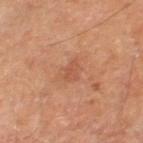follow-up: no biopsy performed (imaged during a skin exam) | TBP lesion metrics: a mean CIELAB color near L≈52 a*≈25 b*≈33 and a normalized lesion–skin contrast near 5; border irregularity of about 3 on a 0–10 scale, internal color variation of about 1.5 on a 0–10 scale, and radial color variation of about 0.5; a nevus-likeness score of about 0/100 | subject: male, aged approximately 70 | site: the right thigh | size: ~2.5 mm (longest diameter) | acquisition: ~15 mm crop, total-body skin-cancer survey.